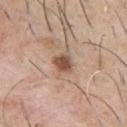No biopsy was performed on this lesion — it was imaged during a full skin examination and was not determined to be concerning. The patient is a male about 60 years old. The recorded lesion diameter is about 2.5 mm. From the chest. A 15 mm crop from a total-body photograph taken for skin-cancer surveillance.